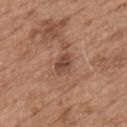Captured during whole-body skin photography for melanoma surveillance; the lesion was not biopsied. The tile uses white-light illumination. About 2.5 mm across. The lesion is located on the chest. Automated image analysis of the tile measured an area of roughly 4 mm², an eccentricity of roughly 0.7, and a symmetry-axis asymmetry near 0.2. The software also gave an average lesion color of about L≈46 a*≈21 b*≈27 (CIELAB), about 10 CIELAB-L* units darker than the surrounding skin, and a lesion-to-skin contrast of about 8 (normalized; higher = more distinct). A 15 mm close-up tile from a total-body photography series done for melanoma screening. A male subject in their mid- to late 60s.